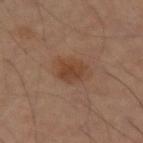No biopsy was performed on this lesion — it was imaged during a full skin examination and was not determined to be concerning.
Cropped from a whole-body photographic skin survey; the tile spans about 15 mm.
From the lower back.
A male patient, about 60 years old.